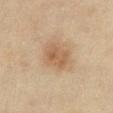{"biopsy_status": "not biopsied; imaged during a skin examination", "site": "chest", "image": {"source": "total-body photography crop", "field_of_view_mm": 15}, "lesion_size": {"long_diameter_mm_approx": 4.0}, "lighting": "cross-polarized", "patient": {"sex": "female", "age_approx": 60}}